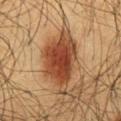| feature | finding |
|---|---|
| lighting | cross-polarized |
| anatomic site | the abdomen |
| patient | male, approximately 60 years of age |
| TBP lesion metrics | two-axis asymmetry of about 0.35; a color-variation rating of about 5.5/10; a nevus-likeness score of about 100/100 and a detector confidence of about 100 out of 100 that the crop contains a lesion |
| imaging modality | 15 mm crop, total-body photography |